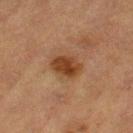Background:
A male patient aged 83 or over. This is a cross-polarized tile. The lesion-visualizer software estimated roughly 11 lightness units darker than nearby skin and a normalized lesion–skin contrast near 10. The software also gave a border-irregularity rating of about 1.5/10 and a color-variation rating of about 3.5/10. Approximately 3.5 mm at its widest. The lesion is on the left thigh. A 15 mm close-up extracted from a 3D total-body photography capture.